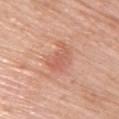{"biopsy_status": "not biopsied; imaged during a skin examination", "site": "upper back", "lighting": "white-light", "patient": {"sex": "female", "age_approx": 65}, "image": {"source": "total-body photography crop", "field_of_view_mm": 15}, "automated_metrics": {"area_mm2_approx": 5.5, "eccentricity": 0.8, "shape_asymmetry": 0.35, "cielab_L": 60, "cielab_a": 26, "cielab_b": 31, "vs_skin_darker_L": 8.0, "vs_skin_contrast_norm": 5.0}, "lesion_size": {"long_diameter_mm_approx": 3.5}}